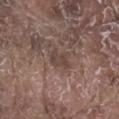Assessment: Recorded during total-body skin imaging; not selected for excision or biopsy. Image and clinical context: Automated image analysis of the tile measured a footprint of about 5 mm² and a symmetry-axis asymmetry near 0.3. And it measured border irregularity of about 3.5 on a 0–10 scale and a within-lesion color-variation index near 2.5/10. The analysis additionally found a detector confidence of about 65 out of 100 that the crop contains a lesion. Approximately 3 mm at its widest. A 15 mm crop from a total-body photograph taken for skin-cancer surveillance. The lesion is on the right lower leg. A male patient aged 78–82.